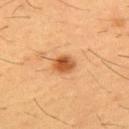Recorded during total-body skin imaging; not selected for excision or biopsy. From the chest. A male subject about 55 years old. A 15 mm close-up extracted from a 3D total-body photography capture.